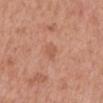| feature | finding |
|---|---|
| image | total-body-photography crop, ~15 mm field of view |
| subject | male, roughly 70 years of age |
| site | the back |
| lesion diameter | ~3 mm (longest diameter) |
| tile lighting | white-light illumination |